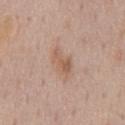Part of a total-body skin-imaging series; this lesion was reviewed on a skin check and was not flagged for biopsy. A roughly 15 mm field-of-view crop from a total-body skin photograph. Imaged with white-light lighting. A male subject, aged 58 to 62. Located on the chest. Approximately 4 mm at its widest.